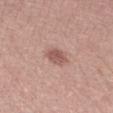Impression: Imaged during a routine full-body skin examination; the lesion was not biopsied and no histopathology is available. Clinical summary: This image is a 15 mm lesion crop taken from a total-body photograph. Captured under white-light illumination. Longest diameter approximately 3 mm. The subject is a male roughly 30 years of age. The total-body-photography lesion software estimated a mean CIELAB color near L≈55 a*≈22 b*≈24, roughly 11 lightness units darker than nearby skin, and a normalized lesion–skin contrast near 7. And it measured a border-irregularity index near 1.5/10 and internal color variation of about 2.5 on a 0–10 scale.